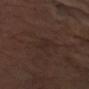Q: Who is the patient?
A: female, aged approximately 65
Q: How was the tile lit?
A: cross-polarized illumination
Q: What kind of image is this?
A: ~15 mm crop, total-body skin-cancer survey
Q: Where on the body is the lesion?
A: the left forearm
Q: What is the lesion's diameter?
A: about 3 mm